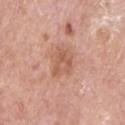Assessment:
The lesion was tiled from a total-body skin photograph and was not biopsied.